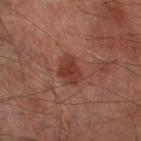<case>
<biopsy_status>not biopsied; imaged during a skin examination</biopsy_status>
<lesion_size>
  <long_diameter_mm_approx>3.5</long_diameter_mm_approx>
</lesion_size>
<patient>
  <sex>male</sex>
  <age_approx>70</age_approx>
</patient>
<automated_metrics>
  <area_mm2_approx>6.5</area_mm2_approx>
  <eccentricity>0.7</eccentricity>
  <shape_asymmetry>0.3</shape_asymmetry>
  <cielab_L>37</cielab_L>
  <cielab_a>24</cielab_a>
  <cielab_b>28</cielab_b>
  <border_irregularity_0_10>3.0</border_irregularity_0_10>
  <color_variation_0_10>2.5</color_variation_0_10>
  <peripheral_color_asymmetry>1.0</peripheral_color_asymmetry>
  <lesion_detection_confidence_0_100>100</lesion_detection_confidence_0_100>
</automated_metrics>
<site>right lower leg</site>
<image>
  <source>total-body photography crop</source>
  <field_of_view_mm>15</field_of_view_mm>
</image>
<lighting>cross-polarized</lighting>
</case>